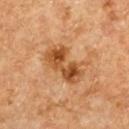The lesion was tiled from a total-body skin photograph and was not biopsied. This is a cross-polarized tile. Longest diameter approximately 5 mm. A female patient in their 60s. This image is a 15 mm lesion crop taken from a total-body photograph. The lesion-visualizer software estimated a lesion area of about 12 mm², a shape eccentricity near 0.85, and a shape-asymmetry score of about 0.25 (0 = symmetric). The software also gave a lesion color around L≈48 a*≈23 b*≈39 in CIELAB and a lesion-to-skin contrast of about 9 (normalized; higher = more distinct). The lesion is on the upper back.